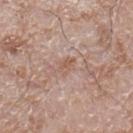Q: Was this lesion biopsied?
A: catalogued during a skin exam; not biopsied
Q: Where on the body is the lesion?
A: the right lower leg
Q: How was this image acquired?
A: total-body-photography crop, ~15 mm field of view
Q: Automated lesion metrics?
A: a lesion area of about 3 mm² and two-axis asymmetry of about 0.4; a mean CIELAB color near L≈57 a*≈18 b*≈28, a lesion–skin lightness drop of about 7, and a lesion-to-skin contrast of about 5.5 (normalized; higher = more distinct); peripheral color asymmetry of about 0.5; a classifier nevus-likeness of about 0/100 and lesion-presence confidence of about 100/100
Q: What lighting was used for the tile?
A: white-light
Q: Patient demographics?
A: male, aged around 60
Q: How large is the lesion?
A: ≈2.5 mm